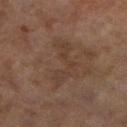Assessment:
The lesion was tiled from a total-body skin photograph and was not biopsied.
Acquisition and patient details:
From the left lower leg. A roughly 15 mm field-of-view crop from a total-body skin photograph. The patient is a female roughly 60 years of age. Approximately 6.5 mm at its widest. Automated image analysis of the tile measured an average lesion color of about L≈33 a*≈14 b*≈23 (CIELAB), roughly 5 lightness units darker than nearby skin, and a normalized border contrast of about 5. The analysis additionally found a border-irregularity rating of about 9.5/10 and a peripheral color-asymmetry measure near 0.5.